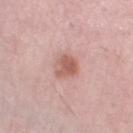Impression: No biopsy was performed on this lesion — it was imaged during a full skin examination and was not determined to be concerning. Background: This image is a 15 mm lesion crop taken from a total-body photograph. A female patient, in their mid-60s. From the left thigh. Automated tile analysis of the lesion measured an area of roughly 6.5 mm² and a shape-asymmetry score of about 0.2 (0 = symmetric). And it measured a mean CIELAB color near L≈59 a*≈23 b*≈26, a lesion–skin lightness drop of about 11, and a normalized border contrast of about 7.5. The analysis additionally found a border-irregularity index near 2/10, a within-lesion color-variation index near 4/10, and peripheral color asymmetry of about 1.5. And it measured an automated nevus-likeness rating near 40 out of 100. Approximately 3.5 mm at its widest. This is a white-light tile.